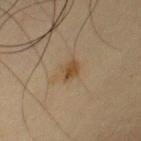{
  "biopsy_status": "not biopsied; imaged during a skin examination",
  "lesion_size": {
    "long_diameter_mm_approx": 3.0
  },
  "site": "chest",
  "lighting": "cross-polarized",
  "patient": {
    "sex": "male",
    "age_approx": 35
  },
  "image": {
    "source": "total-body photography crop",
    "field_of_view_mm": 15
  }
}